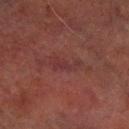Assessment: Recorded during total-body skin imaging; not selected for excision or biopsy.